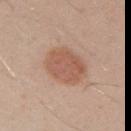The lesion was tiled from a total-body skin photograph and was not biopsied.
Located on the right upper arm.
Automated tile analysis of the lesion measured a lesion color around L≈56 a*≈21 b*≈29 in CIELAB, a lesion–skin lightness drop of about 9, and a normalized border contrast of about 7. It also reported a border-irregularity rating of about 1/10, a color-variation rating of about 3.5/10, and radial color variation of about 1.
A 15 mm close-up tile from a total-body photography series done for melanoma screening.
About 4.5 mm across.
The patient is a male approximately 30 years of age.